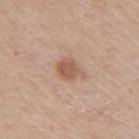This lesion was catalogued during total-body skin photography and was not selected for biopsy.
A 15 mm close-up extracted from a 3D total-body photography capture.
A male subject aged 58–62.
Automated tile analysis of the lesion measured a border-irregularity index near 2/10 and a within-lesion color-variation index near 3/10.
The lesion is located on the right upper arm.